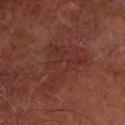Assessment:
The lesion was photographed on a routine skin check and not biopsied; there is no pathology result.
Acquisition and patient details:
The lesion is on the left forearm. The subject is a male aged approximately 65. Imaged with cross-polarized lighting. A 15 mm close-up extracted from a 3D total-body photography capture.